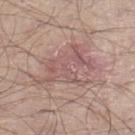Impression:
The lesion was tiled from a total-body skin photograph and was not biopsied.
Background:
The recorded lesion diameter is about 7 mm. A 15 mm close-up tile from a total-body photography series done for melanoma screening. The subject is a male aged 53–57. The tile uses white-light illumination. The lesion is on the left lower leg.